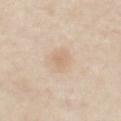Notes:
* notes: imaged on a skin check; not biopsied
* imaging modality: total-body-photography crop, ~15 mm field of view
* location: the front of the torso
* patient: male, aged 58 to 62